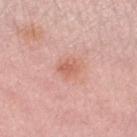Q: Was a biopsy performed?
A: no biopsy performed (imaged during a skin exam)
Q: How was this image acquired?
A: ~15 mm tile from a whole-body skin photo
Q: Illumination type?
A: white-light
Q: What are the patient's age and sex?
A: female, aged around 65
Q: What is the anatomic site?
A: the leg